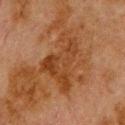biopsy_status: not biopsied; imaged during a skin examination
automated_metrics:
  eccentricity: 0.8
site: upper back
patient:
  sex: male
  age_approx: 80
image:
  source: total-body photography crop
  field_of_view_mm: 15